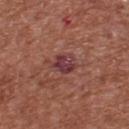Imaged during a routine full-body skin examination; the lesion was not biopsied and no histopathology is available.
Approximately 3 mm at its widest.
From the upper back.
Automated tile analysis of the lesion measured a footprint of about 5.5 mm², an eccentricity of roughly 0.55, and a symmetry-axis asymmetry near 0.2. The software also gave a border-irregularity rating of about 2/10.
This image is a 15 mm lesion crop taken from a total-body photograph.
The tile uses white-light illumination.
A male subject, in their mid- to late 60s.